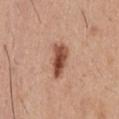Part of a total-body skin-imaging series; this lesion was reviewed on a skin check and was not flagged for biopsy.
On the chest.
The patient is a male aged 28 to 32.
A region of skin cropped from a whole-body photographic capture, roughly 15 mm wide.
Automated image analysis of the tile measured a mean CIELAB color near L≈50 a*≈24 b*≈30, about 16 CIELAB-L* units darker than the surrounding skin, and a normalized lesion–skin contrast near 10.5. It also reported internal color variation of about 6.5 on a 0–10 scale and radial color variation of about 2.5. And it measured an automated nevus-likeness rating near 90 out of 100 and a detector confidence of about 100 out of 100 that the crop contains a lesion.